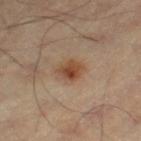The lesion was photographed on a routine skin check and not biopsied; there is no pathology result. The lesion is located on the left thigh. A male subject, about 55 years old. A 15 mm close-up extracted from a 3D total-body photography capture.